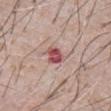follow-up — imaged on a skin check; not biopsied | diameter — about 3 mm | body site — the abdomen | patient — male, aged around 60 | image — total-body-photography crop, ~15 mm field of view | automated lesion analysis — a shape eccentricity near 0.75 and two-axis asymmetry of about 0.3; a mean CIELAB color near L≈52 a*≈28 b*≈21, a lesion–skin lightness drop of about 14, and a normalized lesion–skin contrast near 9.5; a border-irregularity index near 3/10, a within-lesion color-variation index near 4/10, and a peripheral color-asymmetry measure near 1; an automated nevus-likeness rating near 0 out of 100 and lesion-presence confidence of about 100/100.